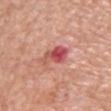diameter: ~3.5 mm (longest diameter) | imaging modality: ~15 mm tile from a whole-body skin photo | subject: male, roughly 60 years of age | location: the chest.